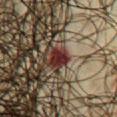Notes:
- follow-up · catalogued during a skin exam; not biopsied
- patient · male, approximately 65 years of age
- image · ~15 mm tile from a whole-body skin photo
- body site · the chest